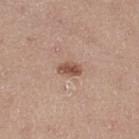| feature | finding |
|---|---|
| biopsy status | catalogued during a skin exam; not biopsied |
| illumination | white-light |
| subject | female, roughly 45 years of age |
| diameter | ~2.5 mm (longest diameter) |
| acquisition | ~15 mm tile from a whole-body skin photo |
| anatomic site | the right thigh |
| TBP lesion metrics | a lesion–skin lightness drop of about 12 and a normalized border contrast of about 8.5; a within-lesion color-variation index near 2/10 and peripheral color asymmetry of about 0.5; a nevus-likeness score of about 90/100 |